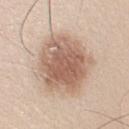{
  "biopsy_status": "not biopsied; imaged during a skin examination",
  "lighting": "white-light",
  "lesion_size": {
    "long_diameter_mm_approx": 7.5
  },
  "site": "right upper arm",
  "image": {
    "source": "total-body photography crop",
    "field_of_view_mm": 15
  },
  "patient": {
    "sex": "male",
    "age_approx": 25
  },
  "automated_metrics": {
    "cielab_L": 61,
    "cielab_a": 17,
    "cielab_b": 28,
    "vs_skin_darker_L": 13.0,
    "vs_skin_contrast_norm": 8.5
  }
}